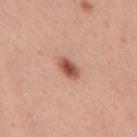follow-up — no biopsy performed (imaged during a skin exam) | anatomic site — the mid back | subject — female, approximately 35 years of age | lesion diameter — ~3 mm (longest diameter) | TBP lesion metrics — an area of roughly 4.5 mm² and an eccentricity of roughly 0.85; an average lesion color of about L≈54 a*≈25 b*≈30 (CIELAB), a lesion–skin lightness drop of about 14, and a normalized lesion–skin contrast near 9.5; an automated nevus-likeness rating near 100 out of 100 and a detector confidence of about 100 out of 100 that the crop contains a lesion | image — total-body-photography crop, ~15 mm field of view.